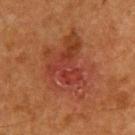Clinical impression:
Captured during whole-body skin photography for melanoma surveillance; the lesion was not biopsied.
Clinical summary:
The lesion's longest dimension is about 7.5 mm. An algorithmic analysis of the crop reported a lesion area of about 31 mm², a shape eccentricity near 0.6, and a symmetry-axis asymmetry near 0.35. And it measured an average lesion color of about L≈34 a*≈25 b*≈28 (CIELAB), roughly 6 lightness units darker than nearby skin, and a lesion-to-skin contrast of about 6.5 (normalized; higher = more distinct). And it measured a border-irregularity rating of about 5.5/10, a color-variation rating of about 5.5/10, and peripheral color asymmetry of about 1.5. It also reported a nevus-likeness score of about 0/100. This is a cross-polarized tile. The lesion is located on the upper back. This image is a 15 mm lesion crop taken from a total-body photograph. A male subject aged around 65.